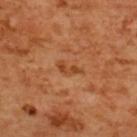• biopsy status — total-body-photography surveillance lesion; no biopsy
• site — the back
• illumination — cross-polarized
• patient — female, aged around 55
• lesion diameter — ≈3 mm
• image source — ~15 mm tile from a whole-body skin photo